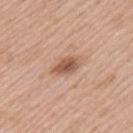Q: Was this lesion biopsied?
A: total-body-photography surveillance lesion; no biopsy
Q: What is the imaging modality?
A: 15 mm crop, total-body photography
Q: Lesion location?
A: the arm
Q: Automated lesion metrics?
A: a footprint of about 5 mm², an outline eccentricity of about 0.85 (0 = round, 1 = elongated), and two-axis asymmetry of about 0.25; about 13 CIELAB-L* units darker than the surrounding skin and a lesion-to-skin contrast of about 8.5 (normalized; higher = more distinct)
Q: Lesion size?
A: ≈3.5 mm
Q: Patient demographics?
A: male, aged around 55
Q: Illumination type?
A: white-light